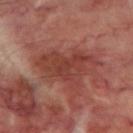A close-up tile cropped from a whole-body skin photograph, about 15 mm across. Automated image analysis of the tile measured a lesion area of about 18 mm² and an eccentricity of roughly 0.8. And it measured a mean CIELAB color near L≈39 a*≈26 b*≈26. The software also gave border irregularity of about 6 on a 0–10 scale, internal color variation of about 4 on a 0–10 scale, and radial color variation of about 1.5. The lesion is on the left thigh. About 7 mm across. A male patient, in their 70s. Captured under cross-polarized illumination.